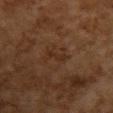| field | value |
|---|---|
| follow-up | total-body-photography surveillance lesion; no biopsy |
| location | the upper back |
| size | ≈3 mm |
| image source | total-body-photography crop, ~15 mm field of view |
| patient | female, aged approximately 60 |